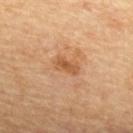  biopsy_status: not biopsied; imaged during a skin examination
  lighting: cross-polarized
  patient:
    sex: male
    age_approx: 85+
  lesion_size:
    long_diameter_mm_approx: 3.0
  image:
    source: total-body photography crop
    field_of_view_mm: 15
  site: upper back